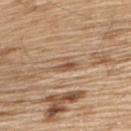workup: no biopsy performed (imaged during a skin exam) | subject: male, aged approximately 70 | lighting: white-light | image source: ~15 mm tile from a whole-body skin photo | anatomic site: the upper back.